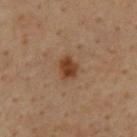Impression:
This lesion was catalogued during total-body skin photography and was not selected for biopsy.
Image and clinical context:
The total-body-photography lesion software estimated an outline eccentricity of about 0.55 (0 = round, 1 = elongated) and a shape-asymmetry score of about 0.2 (0 = symmetric). A male subject, in their mid- to late 60s. A lesion tile, about 15 mm wide, cut from a 3D total-body photograph. The lesion is on the back. Captured under cross-polarized illumination. Approximately 2.5 mm at its widest.